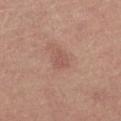Findings:
• follow-up: total-body-photography surveillance lesion; no biopsy
• lesion diameter: ≈2.5 mm
• image: ~15 mm tile from a whole-body skin photo
• location: the left thigh
• patient: female, approximately 65 years of age
• lighting: white-light